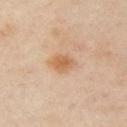| key | value |
|---|---|
| follow-up | catalogued during a skin exam; not biopsied |
| tile lighting | cross-polarized illumination |
| acquisition | ~15 mm tile from a whole-body skin photo |
| image-analysis metrics | a footprint of about 5.5 mm² and two-axis asymmetry of about 0.25; a border-irregularity rating of about 2.5/10 |
| body site | the left arm |
| subject | female, aged 38 to 42 |